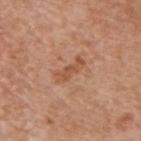<lesion>
<biopsy_status>not biopsied; imaged during a skin examination</biopsy_status>
<image>
  <source>total-body photography crop</source>
  <field_of_view_mm>15</field_of_view_mm>
</image>
<patient>
  <sex>male</sex>
  <age_approx>60</age_approx>
</patient>
<site>upper back</site>
</lesion>